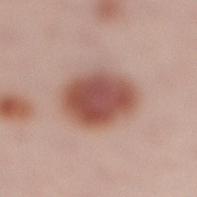No biopsy was performed on this lesion — it was imaged during a full skin examination and was not determined to be concerning. A female subject aged 23 to 27. Measured at roughly 6 mm in maximum diameter. On the right lower leg. Automated tile analysis of the lesion measured a footprint of about 21 mm², a shape eccentricity near 0.7, and a shape-asymmetry score of about 0.15 (0 = symmetric). The software also gave a lesion color around L≈52 a*≈24 b*≈26 in CIELAB and a lesion–skin lightness drop of about 15. It also reported an automated nevus-likeness rating near 100 out of 100 and a lesion-detection confidence of about 100/100. A 15 mm crop from a total-body photograph taken for skin-cancer surveillance.